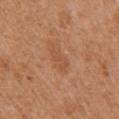The lesion was tiled from a total-body skin photograph and was not biopsied.
This image is a 15 mm lesion crop taken from a total-body photograph.
About 3 mm across.
Located on the chest.
A female subject, roughly 40 years of age.